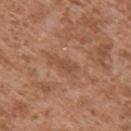Recorded during total-body skin imaging; not selected for excision or biopsy.
A male subject, aged 43–47.
From the right upper arm.
A 15 mm close-up tile from a total-body photography series done for melanoma screening.
Automated image analysis of the tile measured a footprint of about 2.5 mm² and two-axis asymmetry of about 0.55. And it measured an average lesion color of about L≈49 a*≈22 b*≈32 (CIELAB), a lesion–skin lightness drop of about 7, and a normalized lesion–skin contrast near 5. It also reported a border-irregularity rating of about 5.5/10, internal color variation of about 0 on a 0–10 scale, and peripheral color asymmetry of about 0.
Approximately 3 mm at its widest.